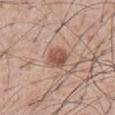follow-up — catalogued during a skin exam; not biopsied
illumination — white-light illumination
subject — male, roughly 55 years of age
lesion size — ≈3 mm
image source — ~15 mm tile from a whole-body skin photo
site — the abdomen